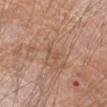Recorded during total-body skin imaging; not selected for excision or biopsy. A male patient aged 63 to 67. A 15 mm crop from a total-body photograph taken for skin-cancer surveillance. This is a white-light tile. The lesion is located on the left forearm. The total-body-photography lesion software estimated a lesion color around L≈53 a*≈19 b*≈30 in CIELAB, a lesion–skin lightness drop of about 6, and a lesion-to-skin contrast of about 4.5 (normalized; higher = more distinct). And it measured a border-irregularity rating of about 5.5/10 and peripheral color asymmetry of about 0. The software also gave an automated nevus-likeness rating near 0 out of 100 and a detector confidence of about 80 out of 100 that the crop contains a lesion.